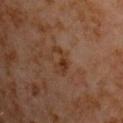Acquisition and patient details: Automated tile analysis of the lesion measured a lesion area of about 5 mm². It also reported an automated nevus-likeness rating near 0 out of 100 and lesion-presence confidence of about 100/100. Longest diameter approximately 3.5 mm. The subject is a male aged approximately 60. The tile uses cross-polarized illumination. A close-up tile cropped from a whole-body skin photograph, about 15 mm across. The lesion is located on the chest.